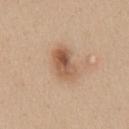Q: Was a biopsy performed?
A: catalogued during a skin exam; not biopsied
Q: What lighting was used for the tile?
A: white-light illumination
Q: How was this image acquired?
A: ~15 mm crop, total-body skin-cancer survey
Q: Patient demographics?
A: male, aged around 35
Q: Lesion location?
A: the abdomen
Q: What is the lesion's diameter?
A: ~4.5 mm (longest diameter)